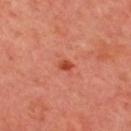biopsy status — catalogued during a skin exam; not biopsied | image — ~15 mm crop, total-body skin-cancer survey | subject — male, approximately 65 years of age | body site — the upper back.